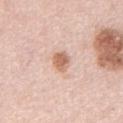notes: total-body-photography surveillance lesion; no biopsy | image source: ~15 mm tile from a whole-body skin photo | subject: male, in their 50s | body site: the chest.